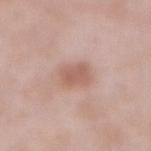The lesion was tiled from a total-body skin photograph and was not biopsied. The lesion is on the front of the torso. This image is a 15 mm lesion crop taken from a total-body photograph. The lesion's longest dimension is about 2.5 mm. A male patient in their mid- to late 50s.